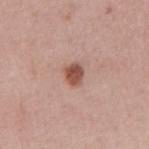Q: Was a biopsy performed?
A: no biopsy performed (imaged during a skin exam)
Q: Where on the body is the lesion?
A: the right upper arm
Q: What is the imaging modality?
A: ~15 mm tile from a whole-body skin photo
Q: Patient demographics?
A: male, aged approximately 60
Q: What lighting was used for the tile?
A: white-light illumination
Q: What is the lesion's diameter?
A: about 2.5 mm